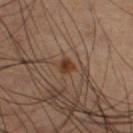No biopsy was performed on this lesion — it was imaged during a full skin examination and was not determined to be concerning.